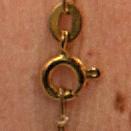Clinical impression: Part of a total-body skin-imaging series; this lesion was reviewed on a skin check and was not flagged for biopsy. Background: The subject is a female about 40 years old. Captured under cross-polarized illumination. Automated image analysis of the tile measured an area of roughly 49 mm², a shape eccentricity near 0.9, and a symmetry-axis asymmetry near 0.55. It also reported a border-irregularity rating of about 8.5/10, a within-lesion color-variation index near 10/10, and a peripheral color-asymmetry measure near 4.5. Longest diameter approximately 13.5 mm. A roughly 15 mm field-of-view crop from a total-body skin photograph. From the head or neck.